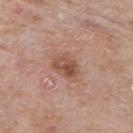Q: Was a biopsy performed?
A: catalogued during a skin exam; not biopsied
Q: Where on the body is the lesion?
A: the upper back
Q: How large is the lesion?
A: ~3.5 mm (longest diameter)
Q: How was this image acquired?
A: 15 mm crop, total-body photography
Q: What lighting was used for the tile?
A: white-light illumination
Q: Who is the patient?
A: male, aged 73 to 77
Q: Automated lesion metrics?
A: a footprint of about 6.5 mm², an outline eccentricity of about 0.65 (0 = round, 1 = elongated), and two-axis asymmetry of about 0.2; a border-irregularity index near 2/10, a color-variation rating of about 5/10, and a peripheral color-asymmetry measure near 2; a nevus-likeness score of about 25/100 and lesion-presence confidence of about 100/100